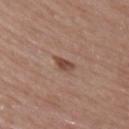Imaged during a routine full-body skin examination; the lesion was not biopsied and no histopathology is available. Captured under white-light illumination. A female patient, approximately 65 years of age. A lesion tile, about 15 mm wide, cut from a 3D total-body photograph. The lesion is on the upper back. An algorithmic analysis of the crop reported a lesion area of about 3 mm², an outline eccentricity of about 0.8 (0 = round, 1 = elongated), and two-axis asymmetry of about 0.4. And it measured a color-variation rating of about 2.5/10. It also reported a classifier nevus-likeness of about 75/100 and a lesion-detection confidence of about 100/100.